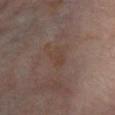This lesion was catalogued during total-body skin photography and was not selected for biopsy.
A region of skin cropped from a whole-body photographic capture, roughly 15 mm wide.
On the leg.
Approximately 2.5 mm at its widest.
The subject is a male about 70 years old.
Captured under cross-polarized illumination.